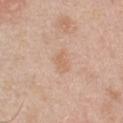Q: Automated lesion metrics?
A: a lesion color around L≈64 a*≈19 b*≈32 in CIELAB; a classifier nevus-likeness of about 0/100 and lesion-presence confidence of about 100/100
Q: Lesion size?
A: about 2.5 mm
Q: How was the tile lit?
A: white-light
Q: Where on the body is the lesion?
A: the chest
Q: What kind of image is this?
A: ~15 mm tile from a whole-body skin photo
Q: Who is the patient?
A: male, roughly 45 years of age